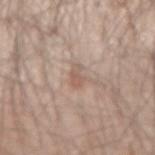Recorded during total-body skin imaging; not selected for excision or biopsy.
About 2.5 mm across.
The patient is a male in their mid- to late 40s.
A 15 mm crop from a total-body photograph taken for skin-cancer surveillance.
Automated image analysis of the tile measured a footprint of about 2.5 mm², an outline eccentricity of about 0.85 (0 = round, 1 = elongated), and two-axis asymmetry of about 0.4. The software also gave an automated nevus-likeness rating near 0 out of 100 and a lesion-detection confidence of about 100/100.
From the right forearm.